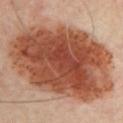| field | value |
|---|---|
| notes | imaged on a skin check; not biopsied |
| illumination | cross-polarized illumination |
| size | ~14.5 mm (longest diameter) |
| anatomic site | the chest |
| patient | male, in their mid- to late 50s |
| acquisition | ~15 mm tile from a whole-body skin photo |